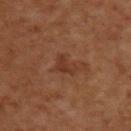No biopsy was performed on this lesion — it was imaged during a full skin examination and was not determined to be concerning. Captured under cross-polarized illumination. Cropped from a total-body skin-imaging series; the visible field is about 15 mm. Measured at roughly 2.5 mm in maximum diameter. A male subject aged 58–62. Automated tile analysis of the lesion measured an area of roughly 4 mm² and an outline eccentricity of about 0.7 (0 = round, 1 = elongated). And it measured a normalized lesion–skin contrast near 6. The software also gave border irregularity of about 4.5 on a 0–10 scale, internal color variation of about 1 on a 0–10 scale, and a peripheral color-asymmetry measure near 0.5. The software also gave a classifier nevus-likeness of about 0/100 and a detector confidence of about 100 out of 100 that the crop contains a lesion. The lesion is located on the upper back.